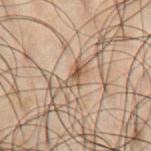biopsy_status: not biopsied; imaged during a skin examination
lesion_size:
  long_diameter_mm_approx: 2.5
site: chest
lighting: cross-polarized
image:
  source: total-body photography crop
  field_of_view_mm: 15
patient:
  sex: male
  age_approx: 50